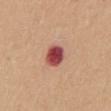{
  "biopsy_status": "not biopsied; imaged during a skin examination",
  "patient": {
    "sex": "male",
    "age_approx": 40
  },
  "lesion_size": {
    "long_diameter_mm_approx": 3.0
  },
  "site": "back",
  "image": {
    "source": "total-body photography crop",
    "field_of_view_mm": 15
  },
  "lighting": "white-light"
}